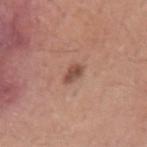workup = imaged on a skin check; not biopsied
acquisition = 15 mm crop, total-body photography
patient = male, aged approximately 30
location = the left upper arm